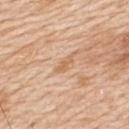biopsy status — imaged on a skin check; not biopsied | TBP lesion metrics — an area of roughly 2 mm², an eccentricity of roughly 0.9, and a symmetry-axis asymmetry near 0.35; an average lesion color of about L≈64 a*≈20 b*≈36 (CIELAB) and a normalized border contrast of about 6; radial color variation of about 0; a lesion-detection confidence of about 100/100 | lesion diameter — ~2.5 mm (longest diameter) | location — the upper back | subject — male, aged 58 to 62 | lighting — white-light illumination | imaging modality — 15 mm crop, total-body photography.